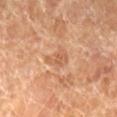biopsy status: no biopsy performed (imaged during a skin exam)
acquisition: ~15 mm tile from a whole-body skin photo
subject: female, aged approximately 70
illumination: cross-polarized illumination
location: the left lower leg